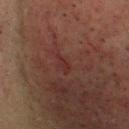Assessment: The lesion was tiled from a total-body skin photograph and was not biopsied. Image and clinical context: The tile uses cross-polarized illumination. The recorded lesion diameter is about 2.5 mm. The patient is a male aged 43–47. Automated image analysis of the tile measured a nevus-likeness score of about 0/100 and a lesion-detection confidence of about 75/100. Located on the head or neck. A 15 mm crop from a total-body photograph taken for skin-cancer surveillance.